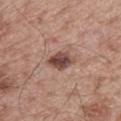Impression: The lesion was photographed on a routine skin check and not biopsied; there is no pathology result.